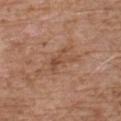This lesion was catalogued during total-body skin photography and was not selected for biopsy.